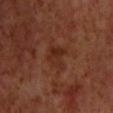Captured during whole-body skin photography for melanoma surveillance; the lesion was not biopsied. Longest diameter approximately 3.5 mm. On the front of the torso. Captured under cross-polarized illumination. A male patient, roughly 70 years of age. A roughly 15 mm field-of-view crop from a total-body skin photograph.